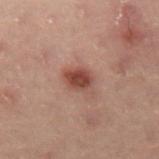Assessment:
No biopsy was performed on this lesion — it was imaged during a full skin examination and was not determined to be concerning.
Acquisition and patient details:
Longest diameter approximately 3 mm. A female patient, about 55 years old. A 15 mm close-up tile from a total-body photography series done for melanoma screening. On the lower back.